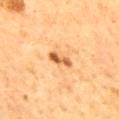Findings:
- notes · imaged on a skin check; not biopsied
- subject · male, aged 58–62
- image-analysis metrics · a footprint of about 3.5 mm², a shape eccentricity near 0.9, and two-axis asymmetry of about 0.3; a mean CIELAB color near L≈61 a*≈26 b*≈45 and a normalized lesion–skin contrast near 9.5; a border-irregularity index near 3/10, a color-variation rating of about 2/10, and a peripheral color-asymmetry measure near 0; a nevus-likeness score of about 45/100 and a lesion-detection confidence of about 100/100
- size · about 3 mm
- image · ~15 mm crop, total-body skin-cancer survey
- illumination · cross-polarized
- anatomic site · the mid back